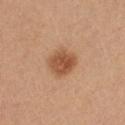The lesion was tiled from a total-body skin photograph and was not biopsied. The lesion's longest dimension is about 3.5 mm. A female patient aged around 25. A region of skin cropped from a whole-body photographic capture, roughly 15 mm wide. The lesion is on the front of the torso.